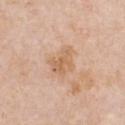Case summary:
* workup — imaged on a skin check; not biopsied
* illumination — white-light illumination
* automated metrics — a mean CIELAB color near L≈63 a*≈19 b*≈35, about 8 CIELAB-L* units darker than the surrounding skin, and a normalized border contrast of about 6; a border-irregularity index near 3.5/10, internal color variation of about 3 on a 0–10 scale, and radial color variation of about 1
* patient — female, approximately 45 years of age
* acquisition — 15 mm crop, total-body photography
* anatomic site — the chest